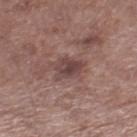Q: Was a biopsy performed?
A: imaged on a skin check; not biopsied
Q: What is the imaging modality?
A: ~15 mm crop, total-body skin-cancer survey
Q: What are the patient's age and sex?
A: male, aged 68–72
Q: What is the anatomic site?
A: the right lower leg
Q: What is the lesion's diameter?
A: ≈3.5 mm